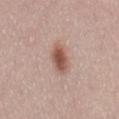notes: total-body-photography surveillance lesion; no biopsy
patient: female, aged 48 to 52
site: the back
illumination: white-light illumination
size: ~4 mm (longest diameter)
acquisition: 15 mm crop, total-body photography
automated metrics: an outline eccentricity of about 0.85 (0 = round, 1 = elongated) and two-axis asymmetry of about 0.2; a mean CIELAB color near L≈54 a*≈21 b*≈26, a lesion–skin lightness drop of about 13, and a lesion-to-skin contrast of about 9 (normalized; higher = more distinct)